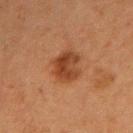biopsy status: catalogued during a skin exam; not biopsied | subject: female, aged 58 to 62 | lesion size: about 4 mm | image source: total-body-photography crop, ~15 mm field of view | body site: the left upper arm.